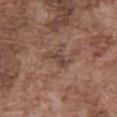Clinical impression: The lesion was photographed on a routine skin check and not biopsied; there is no pathology result. Image and clinical context: On the abdomen. A 15 mm close-up extracted from a 3D total-body photography capture. Longest diameter approximately 3.5 mm. This is a white-light tile. A male subject aged 73 to 77.